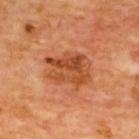Clinical impression:
Part of a total-body skin-imaging series; this lesion was reviewed on a skin check and was not flagged for biopsy.
Image and clinical context:
The total-body-photography lesion software estimated a footprint of about 15 mm², an outline eccentricity of about 0.7 (0 = round, 1 = elongated), and two-axis asymmetry of about 0.25. It also reported a border-irregularity index near 3/10, a color-variation rating of about 6.5/10, and radial color variation of about 2.5. The software also gave a classifier nevus-likeness of about 95/100 and a detector confidence of about 100 out of 100 that the crop contains a lesion. A 15 mm close-up tile from a total-body photography series done for melanoma screening. The lesion is located on the upper back. The subject is a male aged around 60.